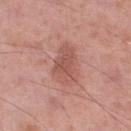Acquisition and patient details:
Approximately 5 mm at its widest. Located on the right lower leg. Cropped from a total-body skin-imaging series; the visible field is about 15 mm. A male patient aged approximately 55. An algorithmic analysis of the crop reported a border-irregularity rating of about 3/10, a color-variation rating of about 3/10, and a peripheral color-asymmetry measure near 1.